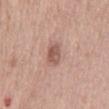The lesion was tiled from a total-body skin photograph and was not biopsied. Longest diameter approximately 3.5 mm. This image is a 15 mm lesion crop taken from a total-body photograph. From the mid back. The patient is a female aged around 60. This is a white-light tile. The total-body-photography lesion software estimated a border-irregularity index near 3/10, a within-lesion color-variation index near 2.5/10, and radial color variation of about 1.